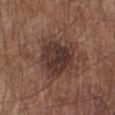biopsy_status: not biopsied; imaged during a skin examination
lesion_size:
  long_diameter_mm_approx: 5.0
patient:
  sex: male
  age_approx: 55
image:
  source: total-body photography crop
  field_of_view_mm: 15
lighting: white-light
site: right lower leg